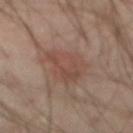{
  "biopsy_status": "not biopsied; imaged during a skin examination",
  "automated_metrics": {
    "cielab_L": 45,
    "cielab_a": 18,
    "cielab_b": 24,
    "vs_skin_darker_L": 6.0,
    "vs_skin_contrast_norm": 5.5,
    "border_irregularity_0_10": 3.5,
    "color_variation_0_10": 3.5,
    "peripheral_color_asymmetry": 1.5
  },
  "image": {
    "source": "total-body photography crop",
    "field_of_view_mm": 15
  },
  "lesion_size": {
    "long_diameter_mm_approx": 4.5
  },
  "lighting": "cross-polarized",
  "site": "abdomen",
  "patient": {
    "sex": "male",
    "age_approx": 65
  }
}